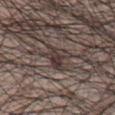The patient is a male in their mid- to late 50s. The lesion is located on the abdomen. Imaged with white-light lighting. Measured at roughly 3 mm in maximum diameter. Cropped from a whole-body photographic skin survey; the tile spans about 15 mm. An algorithmic analysis of the crop reported a footprint of about 5.5 mm², a shape eccentricity near 0.5, and a symmetry-axis asymmetry near 0.4. It also reported a detector confidence of about 55 out of 100 that the crop contains a lesion.